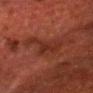This lesion was catalogued during total-body skin photography and was not selected for biopsy.
The lesion is located on the head or neck.
The subject is a male aged around 65.
Cropped from a whole-body photographic skin survey; the tile spans about 15 mm.